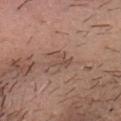{
  "biopsy_status": "not biopsied; imaged during a skin examination",
  "patient": {
    "sex": "male",
    "age_approx": 30
  },
  "site": "head or neck",
  "image": {
    "source": "total-body photography crop",
    "field_of_view_mm": 15
  }
}